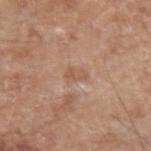Case summary:
– notes: catalogued during a skin exam; not biopsied
– subject: male, in their 60s
– image source: ~15 mm tile from a whole-body skin photo
– TBP lesion metrics: a mean CIELAB color near L≈56 a*≈19 b*≈30, about 6 CIELAB-L* units darker than the surrounding skin, and a normalized lesion–skin contrast near 4.5; an automated nevus-likeness rating near 0 out of 100 and a detector confidence of about 100 out of 100 that the crop contains a lesion
– lesion diameter: ≈2.5 mm
– tile lighting: white-light
– body site: the arm